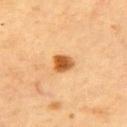No biopsy was performed on this lesion — it was imaged during a full skin examination and was not determined to be concerning. Imaged with cross-polarized lighting. Located on the upper back. This image is a 15 mm lesion crop taken from a total-body photograph. Approximately 2.5 mm at its widest. An algorithmic analysis of the crop reported an average lesion color of about L≈52 a*≈23 b*≈42 (CIELAB) and about 15 CIELAB-L* units darker than the surrounding skin. It also reported an automated nevus-likeness rating near 100 out of 100. A female subject, aged approximately 60.